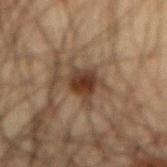• workup — total-body-photography surveillance lesion; no biopsy
• anatomic site — the abdomen
• image — ~15 mm tile from a whole-body skin photo
• size — ≈4 mm
• subject — male, approximately 65 years of age
• automated metrics — roughly 11 lightness units darker than nearby skin and a normalized lesion–skin contrast near 10.5; border irregularity of about 3.5 on a 0–10 scale, a within-lesion color-variation index near 3.5/10, and radial color variation of about 1
• lighting — cross-polarized